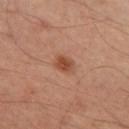Impression:
Captured during whole-body skin photography for melanoma surveillance; the lesion was not biopsied.
Background:
Approximately 2.5 mm at its widest. The total-body-photography lesion software estimated a footprint of about 4 mm² and a shape-asymmetry score of about 0.2 (0 = symmetric). And it measured an average lesion color of about L≈47 a*≈24 b*≈32 (CIELAB). And it measured a border-irregularity rating of about 1.5/10 and a peripheral color-asymmetry measure near 0.5. The analysis additionally found a classifier nevus-likeness of about 90/100. A region of skin cropped from a whole-body photographic capture, roughly 15 mm wide. Located on the left thigh. A male subject about 50 years old.